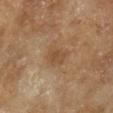Case summary:
• notes — no biopsy performed (imaged during a skin exam)
• lesion diameter — about 3 mm
• patient — male, in their mid- to late 60s
• site — the right lower leg
• image source — total-body-photography crop, ~15 mm field of view